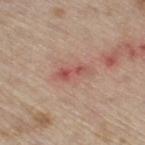Q: Is there a histopathology result?
A: catalogued during a skin exam; not biopsied
Q: Where on the body is the lesion?
A: the right thigh
Q: Patient demographics?
A: male, aged 68–72
Q: Lesion size?
A: ≈4 mm
Q: What is the imaging modality?
A: ~15 mm crop, total-body skin-cancer survey
Q: What lighting was used for the tile?
A: white-light illumination